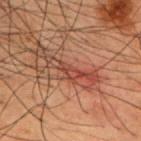{
  "biopsy_status": "not biopsied; imaged during a skin examination",
  "site": "chest",
  "image": {
    "source": "total-body photography crop",
    "field_of_view_mm": 15
  },
  "automated_metrics": {
    "vs_skin_darker_L": 8.0,
    "vs_skin_contrast_norm": 7.0,
    "border_irregularity_0_10": 8.0,
    "color_variation_0_10": 2.5,
    "peripheral_color_asymmetry": 1.0,
    "nevus_likeness_0_100": 20,
    "lesion_detection_confidence_0_100": 60
  },
  "patient": {
    "sex": "male",
    "age_approx": 50
  }
}